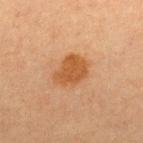workup = no biopsy performed (imaged during a skin exam)
acquisition = 15 mm crop, total-body photography
subject = female, aged 63–67
body site = the back